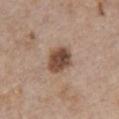<record>
  <biopsy_status>not biopsied; imaged during a skin examination</biopsy_status>
  <image>
    <source>total-body photography crop</source>
    <field_of_view_mm>15</field_of_view_mm>
  </image>
  <site>chest</site>
  <patient>
    <sex>female</sex>
    <age_approx>45</age_approx>
  </patient>
  <lesion_size>
    <long_diameter_mm_approx>3.5</long_diameter_mm_approx>
  </lesion_size>
  <lighting>white-light</lighting>
</record>